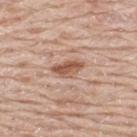{
  "biopsy_status": "not biopsied; imaged during a skin examination",
  "image": {
    "source": "total-body photography crop",
    "field_of_view_mm": 15
  },
  "site": "upper back",
  "patient": {
    "sex": "male",
    "age_approx": 80
  }
}